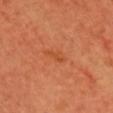biopsy_status: not biopsied; imaged during a skin examination
image:
  source: total-body photography crop
  field_of_view_mm: 15
lighting: cross-polarized
site: chest
patient:
  sex: female
  age_approx: 45